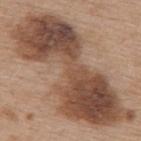Impression:
Part of a total-body skin-imaging series; this lesion was reviewed on a skin check and was not flagged for biopsy.
Image and clinical context:
The tile uses white-light illumination. A 15 mm crop from a total-body photograph taken for skin-cancer surveillance. The lesion is located on the back. Automated tile analysis of the lesion measured a lesion–skin lightness drop of about 15. It also reported an automated nevus-likeness rating near 10 out of 100 and lesion-presence confidence of about 100/100. A female subject, aged 63–67. The recorded lesion diameter is about 16 mm.